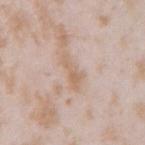notes: catalogued during a skin exam; not biopsied
subject: female, in their mid-20s
illumination: white-light illumination
TBP lesion metrics: internal color variation of about 1 on a 0–10 scale; a nevus-likeness score of about 0/100 and lesion-presence confidence of about 90/100
lesion diameter: about 4 mm
location: the right upper arm
image source: 15 mm crop, total-body photography